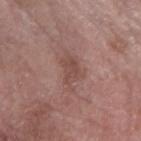patient: male, roughly 75 years of age
site: the arm
lighting: white-light
image: ~15 mm tile from a whole-body skin photo